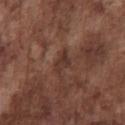Part of a total-body skin-imaging series; this lesion was reviewed on a skin check and was not flagged for biopsy. A roughly 15 mm field-of-view crop from a total-body skin photograph. Located on the chest. A male patient aged approximately 75.